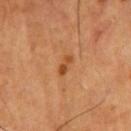* notes — no biopsy performed (imaged during a skin exam)
* subject — male, aged approximately 55
* image source — total-body-photography crop, ~15 mm field of view
* location — the chest